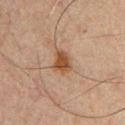biopsy status: imaged on a skin check; not biopsied
tile lighting: cross-polarized illumination
image source: ~15 mm crop, total-body skin-cancer survey
patient: male, roughly 60 years of age
lesion size: ≈3 mm
site: the chest